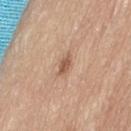biopsy status: total-body-photography surveillance lesion; no biopsy | lesion size: ≈3 mm | illumination: white-light illumination | body site: the back | patient: female, approximately 60 years of age | imaging modality: ~15 mm tile from a whole-body skin photo.